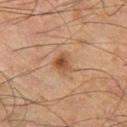Imaged during a routine full-body skin examination; the lesion was not biopsied and no histopathology is available.
The subject is a male aged 63 to 67.
A roughly 15 mm field-of-view crop from a total-body skin photograph.
On the leg.